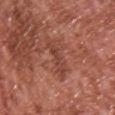patient:
  sex: male
  age_approx: 65
site: upper back
image:
  source: total-body photography crop
  field_of_view_mm: 15